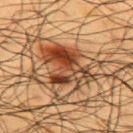Notes:
• workup — imaged on a skin check; not biopsied
• patient — male, aged 58 to 62
• lighting — cross-polarized illumination
• automated lesion analysis — a footprint of about 18 mm², an eccentricity of roughly 0.7, and two-axis asymmetry of about 0.35; a normalized border contrast of about 10.5; a nevus-likeness score of about 60/100
• diameter — ≈6 mm
• body site — the upper back
• acquisition — total-body-photography crop, ~15 mm field of view